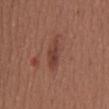Clinical impression:
Recorded during total-body skin imaging; not selected for excision or biopsy.
Clinical summary:
The lesion's longest dimension is about 5 mm. Cropped from a whole-body photographic skin survey; the tile spans about 15 mm. A female patient in their mid-20s. From the mid back. The total-body-photography lesion software estimated a lesion color around L≈41 a*≈23 b*≈26 in CIELAB and a lesion–skin lightness drop of about 8.